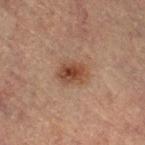follow-up: catalogued during a skin exam; not biopsied | location: the right thigh | illumination: cross-polarized illumination | patient: female, in their 60s | diameter: about 3.5 mm | acquisition: ~15 mm crop, total-body skin-cancer survey.